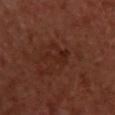Assessment: No biopsy was performed on this lesion — it was imaged during a full skin examination and was not determined to be concerning. Acquisition and patient details: The lesion is on the front of the torso. Imaged with cross-polarized lighting. This image is a 15 mm lesion crop taken from a total-body photograph. The total-body-photography lesion software estimated an area of roughly 4.5 mm², an outline eccentricity of about 0.9 (0 = round, 1 = elongated), and a shape-asymmetry score of about 0.6 (0 = symmetric). The software also gave an average lesion color of about L≈24 a*≈22 b*≈25 (CIELAB), a lesion–skin lightness drop of about 5, and a normalized lesion–skin contrast near 6. And it measured border irregularity of about 7.5 on a 0–10 scale, internal color variation of about 1 on a 0–10 scale, and radial color variation of about 0.5. The software also gave a nevus-likeness score of about 5/100. Longest diameter approximately 4 mm. The patient is a male aged 48–52.